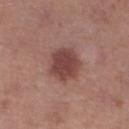  biopsy_status: not biopsied; imaged during a skin examination
  image:
    source: total-body photography crop
    field_of_view_mm: 15
  patient:
    sex: female
    age_approx: 40
  site: left lower leg
  lighting: white-light
  lesion_size:
    long_diameter_mm_approx: 4.5